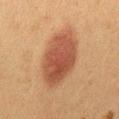Clinical impression: Part of a total-body skin-imaging series; this lesion was reviewed on a skin check and was not flagged for biopsy. Context: The lesion is located on the back. A region of skin cropped from a whole-body photographic capture, roughly 15 mm wide. The recorded lesion diameter is about 7.5 mm. A female patient, aged around 50.